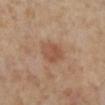Notes:
* follow-up · catalogued during a skin exam; not biopsied
* patient · female, aged around 55
* image · total-body-photography crop, ~15 mm field of view
* size · ≈3.5 mm
* location · the left lower leg
* lighting · cross-polarized